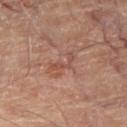* biopsy status: catalogued during a skin exam; not biopsied
* image source: 15 mm crop, total-body photography
* subject: male, in their 70s
* automated lesion analysis: an average lesion color of about L≈48 a*≈23 b*≈26 (CIELAB), a lesion–skin lightness drop of about 7, and a lesion-to-skin contrast of about 5 (normalized; higher = more distinct)
* size: about 3.5 mm
* tile lighting: cross-polarized illumination
* body site: the leg